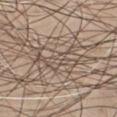{
  "lesion_size": {
    "long_diameter_mm_approx": 7.5
  },
  "lighting": "white-light",
  "site": "front of the torso",
  "patient": {
    "sex": "male",
    "age_approx": 45
  },
  "automated_metrics": {
    "area_mm2_approx": 13.0,
    "eccentricity": 0.95,
    "shape_asymmetry": 0.4,
    "border_irregularity_0_10": 6.0,
    "color_variation_0_10": 5.5,
    "peripheral_color_asymmetry": 2.0
  },
  "image": {
    "source": "total-body photography crop",
    "field_of_view_mm": 15
  }
}